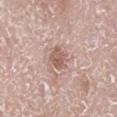Assessment: The lesion was photographed on a routine skin check and not biopsied; there is no pathology result. Acquisition and patient details: A 15 mm crop from a total-body photograph taken for skin-cancer surveillance. A male patient aged 78–82. This is a white-light tile. Measured at roughly 2.5 mm in maximum diameter. On the right lower leg. The lesion-visualizer software estimated internal color variation of about 3.5 on a 0–10 scale and radial color variation of about 1.5. The software also gave an automated nevus-likeness rating near 5 out of 100 and lesion-presence confidence of about 100/100.